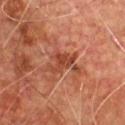The lesion was tiled from a total-body skin photograph and was not biopsied. A lesion tile, about 15 mm wide, cut from a 3D total-body photograph. The subject is a male in their mid-70s. Located on the chest.